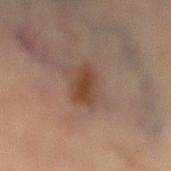Notes:
- notes — catalogued during a skin exam; not biopsied
- image — ~15 mm tile from a whole-body skin photo
- tile lighting — cross-polarized
- image-analysis metrics — a lesion area of about 7.5 mm², a shape eccentricity near 0.7, and a symmetry-axis asymmetry near 0.25; a classifier nevus-likeness of about 90/100 and a detector confidence of about 100 out of 100 that the crop contains a lesion
- body site — the right lower leg
- lesion size — about 3.5 mm
- subject — female, aged approximately 70